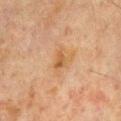workup = total-body-photography surveillance lesion; no biopsy | image = ~15 mm tile from a whole-body skin photo | patient = male, roughly 65 years of age | size = ≈2.5 mm | location = the front of the torso.